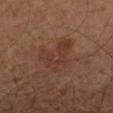The lesion was tiled from a total-body skin photograph and was not biopsied. A male patient aged around 60. Located on the arm. Cropped from a total-body skin-imaging series; the visible field is about 15 mm. The recorded lesion diameter is about 5.5 mm. The lesion-visualizer software estimated a footprint of about 12 mm² and an eccentricity of roughly 0.7. The software also gave about 5 CIELAB-L* units darker than the surrounding skin and a normalized lesion–skin contrast near 5. And it measured border irregularity of about 7 on a 0–10 scale, internal color variation of about 4 on a 0–10 scale, and a peripheral color-asymmetry measure near 1.